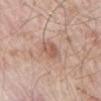Q: Where on the body is the lesion?
A: the mid back
Q: What are the patient's age and sex?
A: male, in their mid-60s
Q: Lesion size?
A: about 3.5 mm
Q: How was this image acquired?
A: ~15 mm crop, total-body skin-cancer survey
Q: Illumination type?
A: white-light illumination